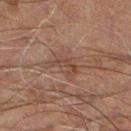Notes:
– workup: no biopsy performed (imaged during a skin exam)
– subject: male, aged around 60
– site: the left lower leg
– image source: total-body-photography crop, ~15 mm field of view
– lesion diameter: ≈3.5 mm
– lighting: cross-polarized